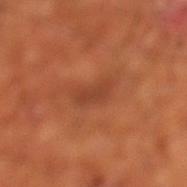This lesion was catalogued during total-body skin photography and was not selected for biopsy.